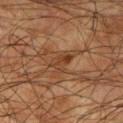Impression:
The lesion was photographed on a routine skin check and not biopsied; there is no pathology result.
Image and clinical context:
Longest diameter approximately 3 mm. A roughly 15 mm field-of-view crop from a total-body skin photograph. The lesion is located on the right upper arm. A male patient aged 73 to 77. This is a cross-polarized tile.